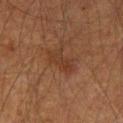The lesion was tiled from a total-body skin photograph and was not biopsied. A male patient, in their mid-60s. From the leg. Imaged with cross-polarized lighting. Automated tile analysis of the lesion measured an average lesion color of about L≈33 a*≈20 b*≈28 (CIELAB), about 6 CIELAB-L* units darker than the surrounding skin, and a lesion-to-skin contrast of about 5.5 (normalized; higher = more distinct). The software also gave a border-irregularity rating of about 3.5/10, a within-lesion color-variation index near 2.5/10, and radial color variation of about 1. The software also gave a nevus-likeness score of about 0/100 and a detector confidence of about 100 out of 100 that the crop contains a lesion. A lesion tile, about 15 mm wide, cut from a 3D total-body photograph. Approximately 4 mm at its widest.